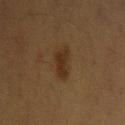notes: catalogued during a skin exam; not biopsied | automated metrics: a lesion area of about 6.5 mm² and a shape-asymmetry score of about 0.3 (0 = symmetric); a mean CIELAB color near L≈28 a*≈14 b*≈28 and a normalized border contrast of about 7.5 | lesion diameter: ~3.5 mm (longest diameter) | anatomic site: the left upper arm | imaging modality: total-body-photography crop, ~15 mm field of view | patient: male, aged approximately 35.